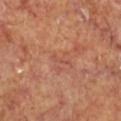No biopsy was performed on this lesion — it was imaged during a full skin examination and was not determined to be concerning. This is a cross-polarized tile. The subject is a male in their mid- to late 60s. Located on the right lower leg. Measured at roughly 2.5 mm in maximum diameter. The total-body-photography lesion software estimated a mean CIELAB color near L≈50 a*≈27 b*≈30, roughly 6 lightness units darker than nearby skin, and a normalized border contrast of about 4.5. Cropped from a whole-body photographic skin survey; the tile spans about 15 mm.